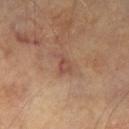Clinical summary: Located on the left thigh. The total-body-photography lesion software estimated a footprint of about 3.5 mm², a shape eccentricity near 0.8, and a symmetry-axis asymmetry near 0.4. The software also gave a lesion color around L≈47 a*≈21 b*≈27 in CIELAB, roughly 7 lightness units darker than nearby skin, and a lesion-to-skin contrast of about 6 (normalized; higher = more distinct). It also reported a nevus-likeness score of about 0/100 and a detector confidence of about 100 out of 100 that the crop contains a lesion. Longest diameter approximately 2.5 mm. A 15 mm close-up tile from a total-body photography series done for melanoma screening. A male subject, aged 68–72. This is a cross-polarized tile.